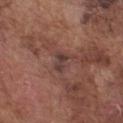biopsy status: no biopsy performed (imaged during a skin exam); patient: male, in their mid-70s; diameter: ≈3 mm; acquisition: ~15 mm crop, total-body skin-cancer survey; anatomic site: the chest.